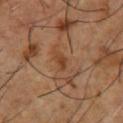Clinical impression:
Recorded during total-body skin imaging; not selected for excision or biopsy.
Image and clinical context:
A male patient aged around 55. A roughly 15 mm field-of-view crop from a total-body skin photograph. The lesion is located on the front of the torso. Approximately 2.5 mm at its widest. This is a cross-polarized tile.